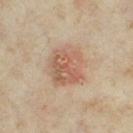Assessment:
Part of a total-body skin-imaging series; this lesion was reviewed on a skin check and was not flagged for biopsy.
Context:
The lesion is located on the right thigh. Automated tile analysis of the lesion measured a border-irregularity index near 1.5/10 and radial color variation of about 2. About 4.5 mm across. Captured under cross-polarized illumination. A female patient in their mid-30s. Cropped from a whole-body photographic skin survey; the tile spans about 15 mm.